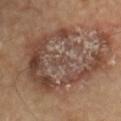Findings:
– follow-up — catalogued during a skin exam; not biopsied
– subject — male, about 65 years old
– lighting — cross-polarized
– lesion size — ~12 mm (longest diameter)
– location — the front of the torso
– image — 15 mm crop, total-body photography